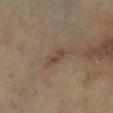Case summary:
- follow-up: no biopsy performed (imaged during a skin exam)
- subject: aged around 60
- body site: the right lower leg
- imaging modality: total-body-photography crop, ~15 mm field of view